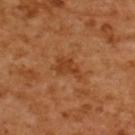The recorded lesion diameter is about 3 mm. On the upper back. Cropped from a whole-body photographic skin survey; the tile spans about 15 mm. The lesion-visualizer software estimated a classifier nevus-likeness of about 0/100 and a detector confidence of about 100 out of 100 that the crop contains a lesion. A female patient, approximately 55 years of age.